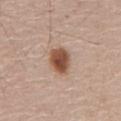The lesion was tiled from a total-body skin photograph and was not biopsied. The lesion's longest dimension is about 3.5 mm. Imaged with white-light lighting. A male subject, aged approximately 60. Located on the front of the torso. A 15 mm close-up extracted from a 3D total-body photography capture.